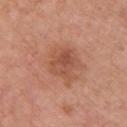Assessment:
Recorded during total-body skin imaging; not selected for excision or biopsy.
Acquisition and patient details:
Located on the upper back. The tile uses white-light illumination. Automated image analysis of the tile measured border irregularity of about 2.5 on a 0–10 scale, internal color variation of about 4 on a 0–10 scale, and a peripheral color-asymmetry measure near 1.5. A lesion tile, about 15 mm wide, cut from a 3D total-body photograph. A female subject, in their 60s.